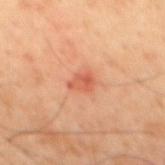Q: Is there a histopathology result?
A: imaged on a skin check; not biopsied